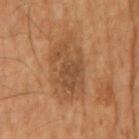– workup — imaged on a skin check; not biopsied
– patient — male, approximately 55 years of age
– image-analysis metrics — an area of roughly 17 mm² and an outline eccentricity of about 0.9 (0 = round, 1 = elongated); a border-irregularity index near 7.5/10, internal color variation of about 3 on a 0–10 scale, and radial color variation of about 1; a classifier nevus-likeness of about 20/100 and lesion-presence confidence of about 100/100
– diameter — ~7 mm (longest diameter)
– lighting — cross-polarized
– imaging modality — total-body-photography crop, ~15 mm field of view
– anatomic site — the mid back